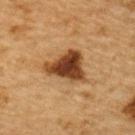Assessment: This lesion was catalogued during total-body skin photography and was not selected for biopsy. Context: A male subject, in their mid- to late 80s or older. From the upper back. A 15 mm close-up extracted from a 3D total-body photography capture.